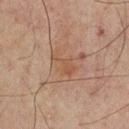Impression:
The lesion was photographed on a routine skin check and not biopsied; there is no pathology result.
Acquisition and patient details:
This is a cross-polarized tile. From the back. The subject is a male in their mid-60s. Measured at roughly 3.5 mm in maximum diameter. A lesion tile, about 15 mm wide, cut from a 3D total-body photograph.